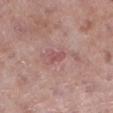Case summary:
* biopsy status: no biopsy performed (imaged during a skin exam)
* image: total-body-photography crop, ~15 mm field of view
* location: the left lower leg
* illumination: white-light illumination
* subject: female, aged approximately 75